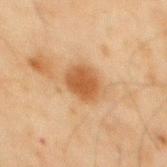– follow-up: imaged on a skin check; not biopsied
– lighting: cross-polarized
– patient: male, aged around 45
– location: the mid back
– automated metrics: a lesion area of about 10 mm², a shape eccentricity near 0.6, and a symmetry-axis asymmetry near 0.15
– image: ~15 mm tile from a whole-body skin photo